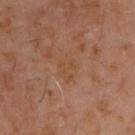notes=no biopsy performed (imaged during a skin exam) | diameter=~3.5 mm (longest diameter) | acquisition=15 mm crop, total-body photography | body site=the upper back | tile lighting=cross-polarized | subject=male, approximately 60 years of age.